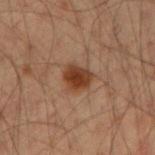{
  "biopsy_status": "not biopsied; imaged during a skin examination",
  "lighting": "cross-polarized",
  "patient": {
    "sex": "male",
    "age_approx": 35
  },
  "site": "right forearm",
  "automated_metrics": {
    "area_mm2_approx": 7.0,
    "shape_asymmetry": 0.2,
    "cielab_L": 36,
    "cielab_a": 20,
    "cielab_b": 29,
    "vs_skin_darker_L": 11.0,
    "vs_skin_contrast_norm": 10.5,
    "lesion_detection_confidence_0_100": 100
  },
  "lesion_size": {
    "long_diameter_mm_approx": 3.0
  },
  "image": {
    "source": "total-body photography crop",
    "field_of_view_mm": 15
  }
}